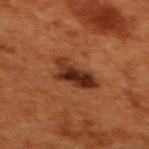• follow-up · imaged on a skin check; not biopsied
• automated metrics · a classifier nevus-likeness of about 80/100
• lighting · cross-polarized illumination
• lesion size · ~5 mm (longest diameter)
• patient · female, aged approximately 50
• anatomic site · the upper back
• acquisition · ~15 mm tile from a whole-body skin photo